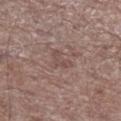Recorded during total-body skin imaging; not selected for excision or biopsy.
About 3 mm across.
On the leg.
This image is a 15 mm lesion crop taken from a total-body photograph.
A male subject, roughly 70 years of age.